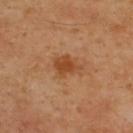biopsy_status: not biopsied; imaged during a skin examination
image:
  source: total-body photography crop
  field_of_view_mm: 15
site: upper back
automated_metrics:
  eccentricity: 0.75
  shape_asymmetry: 0.25
  border_irregularity_0_10: 3.5
lesion_size:
  long_diameter_mm_approx: 3.5
patient:
  sex: male
  age_approx: 45